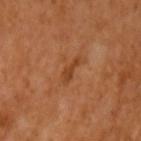notes: imaged on a skin check; not biopsied
image source: total-body-photography crop, ~15 mm field of view
site: the arm
size: ≈3 mm
patient: female, in their mid-50s